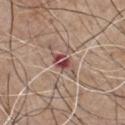This lesion was catalogued during total-body skin photography and was not selected for biopsy. The patient is a male aged around 65. Automated tile analysis of the lesion measured a within-lesion color-variation index near 7/10 and peripheral color asymmetry of about 2.5. A roughly 15 mm field-of-view crop from a total-body skin photograph. This is a white-light tile. The lesion is located on the chest.